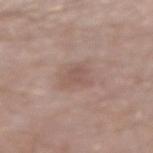follow-up: total-body-photography surveillance lesion; no biopsy
patient: male, in their mid- to late 60s
lesion diameter: about 2.5 mm
image: total-body-photography crop, ~15 mm field of view
body site: the right forearm
TBP lesion metrics: an area of roughly 5 mm², a shape eccentricity near 0.3, and two-axis asymmetry of about 0.4; a lesion color around L≈54 a*≈17 b*≈23 in CIELAB, a lesion–skin lightness drop of about 7, and a normalized border contrast of about 5; a border-irregularity rating of about 4/10 and a color-variation rating of about 1.5/10
lighting: white-light illumination